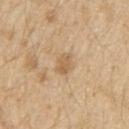* biopsy status: catalogued during a skin exam; not biopsied
* automated lesion analysis: a mean CIELAB color near L≈61 a*≈16 b*≈37, about 9 CIELAB-L* units darker than the surrounding skin, and a lesion-to-skin contrast of about 6 (normalized; higher = more distinct); a border-irregularity index near 2.5/10 and a within-lesion color-variation index near 2/10; a classifier nevus-likeness of about 0/100 and lesion-presence confidence of about 100/100
* lighting: white-light
* patient: male, approximately 70 years of age
* diameter: ~3 mm (longest diameter)
* image: ~15 mm tile from a whole-body skin photo
* location: the arm